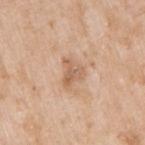biopsy status: imaged on a skin check; not biopsied
anatomic site: the right upper arm
size: ≈3 mm
subject: male, roughly 65 years of age
TBP lesion metrics: a footprint of about 5 mm²; an average lesion color of about L≈61 a*≈20 b*≈32 (CIELAB), a lesion–skin lightness drop of about 9, and a normalized border contrast of about 6
image: total-body-photography crop, ~15 mm field of view
lighting: white-light